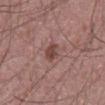The lesion was photographed on a routine skin check and not biopsied; there is no pathology result. The subject is a male roughly 60 years of age. Located on the abdomen. Approximately 3 mm at its widest. This image is a 15 mm lesion crop taken from a total-body photograph. This is a white-light tile.